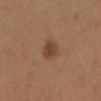Clinical impression: No biopsy was performed on this lesion — it was imaged during a full skin examination and was not determined to be concerning. Background: A female patient about 30 years old. Located on the mid back. Longest diameter approximately 3 mm. A lesion tile, about 15 mm wide, cut from a 3D total-body photograph.